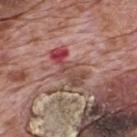Captured during whole-body skin photography for melanoma surveillance; the lesion was not biopsied.